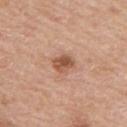| key | value |
|---|---|
| biopsy status | imaged on a skin check; not biopsied |
| imaging modality | 15 mm crop, total-body photography |
| patient | male, roughly 60 years of age |
| site | the back |
| lighting | white-light |
| diameter | ~3 mm (longest diameter) |